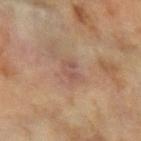Assessment:
Imaged during a routine full-body skin examination; the lesion was not biopsied and no histopathology is available.
Clinical summary:
From the left forearm. A 15 mm close-up tile from a total-body photography series done for melanoma screening. A female patient aged 58–62.